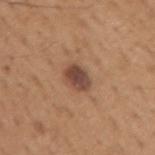Recorded during total-body skin imaging; not selected for excision or biopsy. Cropped from a whole-body photographic skin survey; the tile spans about 15 mm. The patient is a male in their mid-60s. The lesion is on the right upper arm.